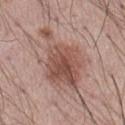<tbp_lesion>
  <biopsy_status>not biopsied; imaged during a skin examination</biopsy_status>
  <lesion_size>
    <long_diameter_mm_approx>8.0</long_diameter_mm_approx>
  </lesion_size>
  <image>
    <source>total-body photography crop</source>
    <field_of_view_mm>15</field_of_view_mm>
  </image>
  <lighting>white-light</lighting>
  <site>abdomen</site>
  <patient>
    <sex>male</sex>
    <age_approx>55</age_approx>
  </patient>
</tbp_lesion>